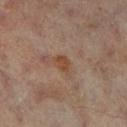biopsy_status: not biopsied; imaged during a skin examination
site: right lower leg
patient:
  sex: male
  age_approx: 65
lesion_size:
  long_diameter_mm_approx: 2.5
image:
  source: total-body photography crop
  field_of_view_mm: 15
lighting: cross-polarized